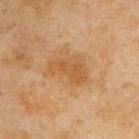Impression: Imaged during a routine full-body skin examination; the lesion was not biopsied and no histopathology is available. Image and clinical context: Captured under cross-polarized illumination. From the back. The total-body-photography lesion software estimated a border-irregularity index near 4.5/10 and radial color variation of about 0.5. The analysis additionally found a nevus-likeness score of about 5/100 and lesion-presence confidence of about 100/100. Approximately 4.5 mm at its widest. A roughly 15 mm field-of-view crop from a total-body skin photograph. A male subject aged 43–47.